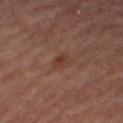Part of a total-body skin-imaging series; this lesion was reviewed on a skin check and was not flagged for biopsy.
Imaged with cross-polarized lighting.
The lesion is on the leg.
A female patient, aged 58 to 62.
This image is a 15 mm lesion crop taken from a total-body photograph.
Measured at roughly 3 mm in maximum diameter.
Automated tile analysis of the lesion measured a border-irregularity rating of about 2.5/10, a color-variation rating of about 3/10, and radial color variation of about 1. It also reported lesion-presence confidence of about 100/100.